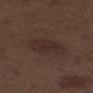Captured during whole-body skin photography for melanoma surveillance; the lesion was not biopsied. A close-up tile cropped from a whole-body skin photograph, about 15 mm across. The patient is a male aged 68–72. The lesion is located on the leg. Approximately 5 mm at its widest.